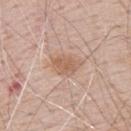biopsy status: total-body-photography surveillance lesion; no biopsy | diameter: ≈3.5 mm | TBP lesion metrics: a shape eccentricity near 0.7; a lesion color around L≈60 a*≈19 b*≈30 in CIELAB and roughly 9 lightness units darker than nearby skin; a border-irregularity index near 2.5/10, internal color variation of about 2 on a 0–10 scale, and a peripheral color-asymmetry measure near 0.5 | image source: ~15 mm tile from a whole-body skin photo | subject: male, aged 68–72 | site: the upper back | illumination: white-light.